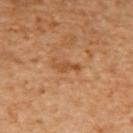Impression:
This lesion was catalogued during total-body skin photography and was not selected for biopsy.
Clinical summary:
A female subject about 55 years old. A 15 mm close-up extracted from a 3D total-body photography capture. Located on the upper back.